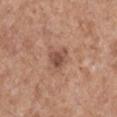Background: The lesion is located on the right upper arm. A 15 mm close-up extracted from a 3D total-body photography capture. Imaged with white-light lighting. A male subject, aged approximately 70. Longest diameter approximately 2.5 mm.